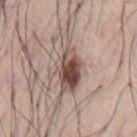Captured during whole-body skin photography for melanoma surveillance; the lesion was not biopsied. A male patient aged 73 to 77. Longest diameter approximately 8 mm. Imaged with white-light lighting. This image is a 15 mm lesion crop taken from a total-body photograph. Automated tile analysis of the lesion measured an area of roughly 15 mm², an eccentricity of roughly 0.9, and a symmetry-axis asymmetry near 0.45. The analysis additionally found a mean CIELAB color near L≈51 a*≈16 b*≈22, about 15 CIELAB-L* units darker than the surrounding skin, and a normalized border contrast of about 10. The software also gave a border-irregularity index near 6.5/10 and internal color variation of about 10 on a 0–10 scale. The lesion is located on the abdomen.